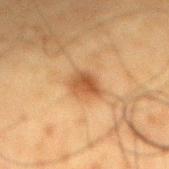{"biopsy_status": "not biopsied; imaged during a skin examination", "lighting": "cross-polarized", "patient": {"sex": "male", "age_approx": 60}, "site": "mid back", "lesion_size": {"long_diameter_mm_approx": 3.0}, "image": {"source": "total-body photography crop", "field_of_view_mm": 15}}